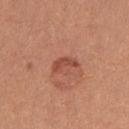Findings:
- biopsy status — catalogued during a skin exam; not biopsied
- location — the left upper arm
- lesion diameter — ≈3 mm
- patient — female, aged approximately 25
- image source — ~15 mm crop, total-body skin-cancer survey
- lighting — white-light
- TBP lesion metrics — a mean CIELAB color near L≈49 a*≈28 b*≈31, a lesion–skin lightness drop of about 9, and a normalized border contrast of about 6.5; a lesion-detection confidence of about 100/100